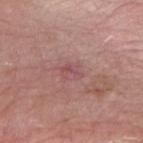The lesion was tiled from a total-body skin photograph and was not biopsied. The lesion is on the right forearm. This is a white-light tile. The recorded lesion diameter is about 2.5 mm. The lesion-visualizer software estimated a mean CIELAB color near L≈51 a*≈25 b*≈20 and a normalized lesion–skin contrast near 5. The analysis additionally found a nevus-likeness score of about 0/100. A male subject, aged around 60. A 15 mm close-up extracted from a 3D total-body photography capture.